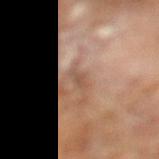Q: Is there a histopathology result?
A: total-body-photography surveillance lesion; no biopsy
Q: Who is the patient?
A: male, aged 68 to 72
Q: What kind of image is this?
A: ~15 mm tile from a whole-body skin photo
Q: How was the tile lit?
A: cross-polarized illumination
Q: What is the anatomic site?
A: the left lower leg
Q: How large is the lesion?
A: ≈3 mm
Q: Automated lesion metrics?
A: a footprint of about 2 mm², a shape eccentricity near 0.95, and a shape-asymmetry score of about 0.4 (0 = symmetric); a border-irregularity index near 4.5/10, internal color variation of about 0 on a 0–10 scale, and a peripheral color-asymmetry measure near 0; an automated nevus-likeness rating near 0 out of 100 and lesion-presence confidence of about 70/100